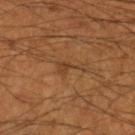Imaged during a routine full-body skin examination; the lesion was not biopsied and no histopathology is available. The patient is a male in their mid- to late 50s. Cropped from a whole-body photographic skin survey; the tile spans about 15 mm. Longest diameter approximately 3.5 mm. From the left forearm. The lesion-visualizer software estimated a lesion color around L≈39 a*≈19 b*≈33 in CIELAB, roughly 6 lightness units darker than nearby skin, and a normalized border contrast of about 5.5. And it measured peripheral color asymmetry of about 0.5. Captured under cross-polarized illumination.